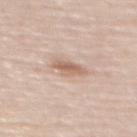notes: catalogued during a skin exam; not biopsied
location: the upper back
subject: female, roughly 60 years of age
lesion diameter: about 3.5 mm
acquisition: total-body-photography crop, ~15 mm field of view
lighting: white-light
automated lesion analysis: a lesion area of about 5.5 mm², an outline eccentricity of about 0.8 (0 = round, 1 = elongated), and a symmetry-axis asymmetry near 0.3; a mean CIELAB color near L≈63 a*≈18 b*≈28 and about 11 CIELAB-L* units darker than the surrounding skin; peripheral color asymmetry of about 1.5; lesion-presence confidence of about 100/100